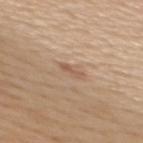Notes:
• notes: catalogued during a skin exam; not biopsied
• subject: female, aged 43 to 47
• diameter: about 2.5 mm
• location: the upper back
• illumination: white-light illumination
• imaging modality: ~15 mm tile from a whole-body skin photo